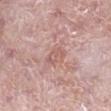Imaged during a routine full-body skin examination; the lesion was not biopsied and no histopathology is available. The recorded lesion diameter is about 3 mm. Located on the leg. Cropped from a whole-body photographic skin survey; the tile spans about 15 mm. The subject is a male aged around 50. This is a white-light tile. Automated tile analysis of the lesion measured a shape eccentricity near 0.75 and two-axis asymmetry of about 0.25. The software also gave a mean CIELAB color near L≈59 a*≈22 b*≈24, about 7 CIELAB-L* units darker than the surrounding skin, and a lesion-to-skin contrast of about 4.5 (normalized; higher = more distinct).